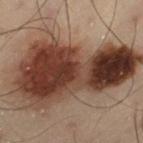Impression: No biopsy was performed on this lesion — it was imaged during a full skin examination and was not determined to be concerning. Acquisition and patient details: A lesion tile, about 15 mm wide, cut from a 3D total-body photograph. About 13 mm across. The lesion-visualizer software estimated an area of roughly 60 mm² and a symmetry-axis asymmetry near 0.35. The analysis additionally found a lesion color around L≈29 a*≈16 b*≈21 in CIELAB, roughly 16 lightness units darker than nearby skin, and a lesion-to-skin contrast of about 14.5 (normalized; higher = more distinct). And it measured a classifier nevus-likeness of about 100/100. The tile uses cross-polarized illumination. The lesion is on the left thigh. A male patient, approximately 55 years of age.